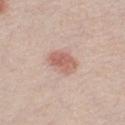Assessment:
Part of a total-body skin-imaging series; this lesion was reviewed on a skin check and was not flagged for biopsy.
Context:
This is a white-light tile. The subject is a male aged 28 to 32. Longest diameter approximately 3.5 mm. This image is a 15 mm lesion crop taken from a total-body photograph.